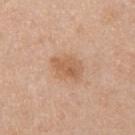- biopsy status · no biopsy performed (imaged during a skin exam)
- site · the upper back
- patient · female, aged 38 to 42
- illumination · white-light
- acquisition · ~15 mm crop, total-body skin-cancer survey